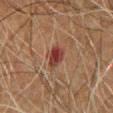This lesion was catalogued during total-body skin photography and was not selected for biopsy. The lesion is located on the chest. A 15 mm close-up extracted from a 3D total-body photography capture. A male patient about 65 years old. About 3.5 mm across. This is a cross-polarized tile.